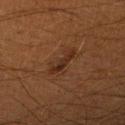Clinical impression:
No biopsy was performed on this lesion — it was imaged during a full skin examination and was not determined to be concerning.
Clinical summary:
A male subject approximately 60 years of age. Approximately 4 mm at its widest. The lesion is on the right lower leg. A 15 mm crop from a total-body photograph taken for skin-cancer surveillance. Imaged with cross-polarized lighting.